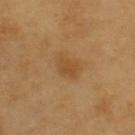biopsy_status: not biopsied; imaged during a skin examination
lesion_size:
  long_diameter_mm_approx: 2.5
image:
  source: total-body photography crop
  field_of_view_mm: 15
site: back
lighting: cross-polarized
patient:
  sex: male
  age_approx: 60
automated_metrics:
  area_mm2_approx: 5.0
  eccentricity: 0.65
  shape_asymmetry: 0.2
  border_irregularity_0_10: 2.0
  color_variation_0_10: 2.0
  peripheral_color_asymmetry: 0.5
  nevus_likeness_0_100: 20
  lesion_detection_confidence_0_100: 100